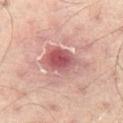| field | value |
|---|---|
| notes | catalogued during a skin exam; not biopsied |
| body site | the left thigh |
| patient | male, approximately 65 years of age |
| acquisition | ~15 mm tile from a whole-body skin photo |
| size | ~5.5 mm (longest diameter) |